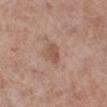<lesion>
<biopsy_status>not biopsied; imaged during a skin examination</biopsy_status>
<automated_metrics>
  <cielab_L>53</cielab_L>
  <cielab_a>20</cielab_a>
  <cielab_b>28</cielab_b>
  <vs_skin_darker_L>9.0</vs_skin_darker_L>
  <vs_skin_contrast_norm>6.5</vs_skin_contrast_norm>
  <border_irregularity_0_10>3.0</border_irregularity_0_10>
  <color_variation_0_10>1.5</color_variation_0_10>
</automated_metrics>
<site>right lower leg</site>
<image>
  <source>total-body photography crop</source>
  <field_of_view_mm>15</field_of_view_mm>
</image>
<lighting>white-light</lighting>
<patient>
  <sex>female</sex>
  <age_approx>50</age_approx>
</patient>
<lesion_size>
  <long_diameter_mm_approx>3.0</long_diameter_mm_approx>
</lesion_size>
</lesion>